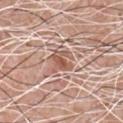notes: imaged on a skin check; not biopsied | automated metrics: a lesion area of about 6.5 mm², an outline eccentricity of about 0.2 (0 = round, 1 = elongated), and a shape-asymmetry score of about 0.35 (0 = symmetric); an average lesion color of about L≈56 a*≈21 b*≈28 (CIELAB), roughly 10 lightness units darker than nearby skin, and a normalized border contrast of about 7; border irregularity of about 5 on a 0–10 scale and peripheral color asymmetry of about 1; a classifier nevus-likeness of about 0/100 and lesion-presence confidence of about 55/100 | illumination: white-light illumination | diameter: ~3 mm (longest diameter) | site: the chest | imaging modality: ~15 mm crop, total-body skin-cancer survey | subject: male, aged 58 to 62.